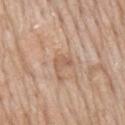Clinical impression:
This lesion was catalogued during total-body skin photography and was not selected for biopsy.
Image and clinical context:
From the mid back. About 3 mm across. A region of skin cropped from a whole-body photographic capture, roughly 15 mm wide. A male patient aged approximately 60. This is a white-light tile.